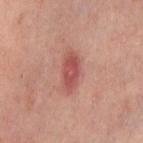Q: Where on the body is the lesion?
A: the right thigh
Q: How was this image acquired?
A: 15 mm crop, total-body photography
Q: Who is the patient?
A: female, about 50 years old
Q: How large is the lesion?
A: ~4.5 mm (longest diameter)